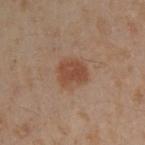Clinical impression:
The lesion was tiled from a total-body skin photograph and was not biopsied.
Acquisition and patient details:
From the left arm. The total-body-photography lesion software estimated an area of roughly 7 mm², an eccentricity of roughly 0.65, and a shape-asymmetry score of about 0.2 (0 = symmetric). It also reported an average lesion color of about L≈36 a*≈18 b*≈25 (CIELAB), a lesion–skin lightness drop of about 8, and a normalized border contrast of about 7.5. Measured at roughly 3.5 mm in maximum diameter. The patient is a male roughly 50 years of age. This image is a 15 mm lesion crop taken from a total-body photograph.